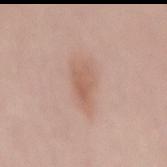Captured during whole-body skin photography for melanoma surveillance; the lesion was not biopsied.
The lesion is located on the mid back.
A female patient, aged approximately 60.
Cropped from a whole-body photographic skin survey; the tile spans about 15 mm.